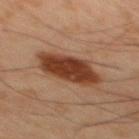Clinical summary:
On the mid back. A 15 mm close-up extracted from a 3D total-body photography capture. The subject is a male roughly 45 years of age.